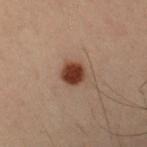biopsy_status: not biopsied; imaged during a skin examination
patient:
  sex: male
  age_approx: 55
site: arm
lesion_size:
  long_diameter_mm_approx: 2.5
image:
  source: total-body photography crop
  field_of_view_mm: 15
lighting: cross-polarized
automated_metrics:
  area_mm2_approx: 6.0
  eccentricity: 0.25
  shape_asymmetry: 0.1
  border_irregularity_0_10: 1.0
  color_variation_0_10: 2.0
  nevus_likeness_0_100: 100
  lesion_detection_confidence_0_100: 100